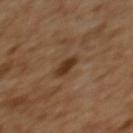The lesion was tiled from a total-body skin photograph and was not biopsied.
A female patient, aged 53 to 57.
The lesion is located on the back.
Imaged with cross-polarized lighting.
Automated image analysis of the tile measured a normalized lesion–skin contrast near 9.5. The software also gave an automated nevus-likeness rating near 75 out of 100 and a detector confidence of about 100 out of 100 that the crop contains a lesion.
Measured at roughly 2.5 mm in maximum diameter.
A close-up tile cropped from a whole-body skin photograph, about 15 mm across.